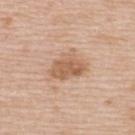Q: Is there a histopathology result?
A: imaged on a skin check; not biopsied
Q: What is the anatomic site?
A: the upper back
Q: Illumination type?
A: white-light illumination
Q: Who is the patient?
A: female, aged approximately 45
Q: What is the lesion's diameter?
A: about 4 mm
Q: What is the imaging modality?
A: total-body-photography crop, ~15 mm field of view